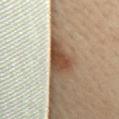Q: Was this lesion biopsied?
A: imaged on a skin check; not biopsied
Q: What is the lesion's diameter?
A: about 4.5 mm
Q: Patient demographics?
A: female, aged around 45
Q: What is the anatomic site?
A: the mid back
Q: How was the tile lit?
A: cross-polarized
Q: How was this image acquired?
A: ~15 mm crop, total-body skin-cancer survey